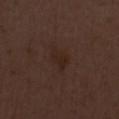- follow-up — imaged on a skin check; not biopsied
- subject — female, aged approximately 50
- body site — the chest
- tile lighting — white-light
- lesion diameter — ~2.5 mm (longest diameter)
- imaging modality — 15 mm crop, total-body photography